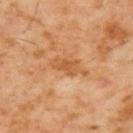The lesion was photographed on a routine skin check and not biopsied; there is no pathology result.
About 4 mm across.
A lesion tile, about 15 mm wide, cut from a 3D total-body photograph.
Automated tile analysis of the lesion measured a mean CIELAB color near L≈52 a*≈22 b*≈39, a lesion–skin lightness drop of about 8, and a normalized lesion–skin contrast near 6.5. It also reported border irregularity of about 3 on a 0–10 scale, a within-lesion color-variation index near 2/10, and a peripheral color-asymmetry measure near 0.5. The software also gave lesion-presence confidence of about 100/100.
A male subject in their 60s.
Captured under cross-polarized illumination.
On the upper back.